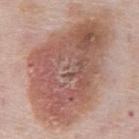follow-up = catalogued during a skin exam; not biopsied
location = the front of the torso
imaging modality = total-body-photography crop, ~15 mm field of view
illumination = white-light illumination
patient = male, roughly 75 years of age
image-analysis metrics = a lesion area of about 70 mm² and a shape eccentricity near 0.8; a mean CIELAB color near L≈55 a*≈21 b*≈26 and roughly 13 lightness units darker than nearby skin; a border-irregularity rating of about 3.5/10, a color-variation rating of about 7.5/10, and radial color variation of about 2.5
diameter = about 13 mm